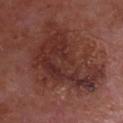biopsy status — catalogued during a skin exam; not biopsied | body site — the chest | TBP lesion metrics — a mean CIELAB color near L≈32 a*≈23 b*≈23, about 9 CIELAB-L* units darker than the surrounding skin, and a normalized border contrast of about 8.5 | image source — total-body-photography crop, ~15 mm field of view | patient — male, aged approximately 65.